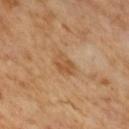Imaged during a routine full-body skin examination; the lesion was not biopsied and no histopathology is available.
A male patient in their mid- to late 60s.
Measured at roughly 3 mm in maximum diameter.
Imaged with cross-polarized lighting.
A 15 mm close-up extracted from a 3D total-body photography capture.